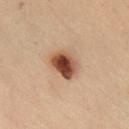This lesion was catalogued during total-body skin photography and was not selected for biopsy.
On the chest.
A female patient, aged around 40.
A roughly 15 mm field-of-view crop from a total-body skin photograph.